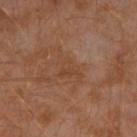biopsy_status: not biopsied; imaged during a skin examination
site: left arm
patient:
  sex: male
  age_approx: 30
image:
  source: total-body photography crop
  field_of_view_mm: 15
lighting: cross-polarized